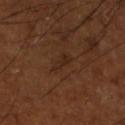{
  "biopsy_status": "not biopsied; imaged during a skin examination",
  "lesion_size": {
    "long_diameter_mm_approx": 3.0
  },
  "patient": {
    "sex": "male",
    "age_approx": 65
  },
  "lighting": "cross-polarized",
  "automated_metrics": {
    "vs_skin_darker_L": 5.0,
    "vs_skin_contrast_norm": 5.5,
    "nevus_likeness_0_100": 0
  },
  "image": {
    "source": "total-body photography crop",
    "field_of_view_mm": 15
  },
  "site": "leg"
}